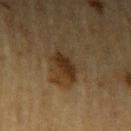workup = no biopsy performed (imaged during a skin exam).